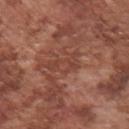Assessment:
Imaged during a routine full-body skin examination; the lesion was not biopsied and no histopathology is available.
Context:
From the arm. The subject is a male aged 73–77. A 15 mm crop from a total-body photograph taken for skin-cancer surveillance. Automated image analysis of the tile measured a border-irregularity rating of about 5.5/10, a color-variation rating of about 2/10, and peripheral color asymmetry of about 0.5. The software also gave an automated nevus-likeness rating near 0 out of 100 and a lesion-detection confidence of about 55/100. The recorded lesion diameter is about 4 mm.